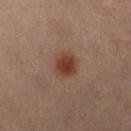follow-up = imaged on a skin check; not biopsied | lighting = cross-polarized | diameter = ≈3 mm | body site = the leg | subject = female, aged approximately 50 | acquisition = ~15 mm tile from a whole-body skin photo.